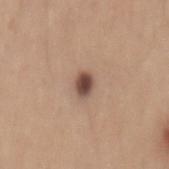Findings:
• biopsy status · catalogued during a skin exam; not biopsied
• location · the mid back
• size · ~2.5 mm (longest diameter)
• image source · ~15 mm tile from a whole-body skin photo
• patient · male, aged 28 to 32